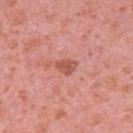notes: catalogued during a skin exam; not biopsied
subject: female, in their 40s
imaging modality: ~15 mm tile from a whole-body skin photo
automated metrics: a shape eccentricity near 0.7 and a shape-asymmetry score of about 0.25 (0 = symmetric); an average lesion color of about L≈55 a*≈29 b*≈31 (CIELAB), a lesion–skin lightness drop of about 10, and a normalized lesion–skin contrast near 7; a detector confidence of about 100 out of 100 that the crop contains a lesion
body site: the upper back
lighting: white-light illumination
lesion size: about 2.5 mm